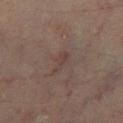Part of a total-body skin-imaging series; this lesion was reviewed on a skin check and was not flagged for biopsy. Cropped from a whole-body photographic skin survey; the tile spans about 15 mm. A male subject about 75 years old. The lesion is on the right lower leg.